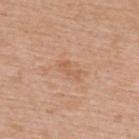biopsy_status: not biopsied; imaged during a skin examination
image:
  source: total-body photography crop
  field_of_view_mm: 15
automated_metrics:
  area_mm2_approx: 3.0
  shape_asymmetry: 0.55
  nevus_likeness_0_100: 0
  lesion_detection_confidence_0_100: 100
patient:
  sex: female
  age_approx: 65
site: back